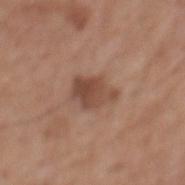Q: Was this lesion biopsied?
A: imaged on a skin check; not biopsied
Q: Automated lesion metrics?
A: a lesion color around L≈47 a*≈20 b*≈27 in CIELAB and a lesion–skin lightness drop of about 10; border irregularity of about 3 on a 0–10 scale, a color-variation rating of about 5/10, and a peripheral color-asymmetry measure near 2
Q: What kind of image is this?
A: ~15 mm tile from a whole-body skin photo
Q: Illumination type?
A: white-light illumination
Q: Where on the body is the lesion?
A: the mid back
Q: Patient demographics?
A: male, in their mid- to late 70s
Q: Lesion size?
A: about 4 mm